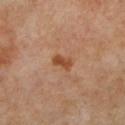Imaged during a routine full-body skin examination; the lesion was not biopsied and no histopathology is available. This is a cross-polarized tile. An algorithmic analysis of the crop reported internal color variation of about 2.5 on a 0–10 scale and a peripheral color-asymmetry measure near 0.5. The software also gave a nevus-likeness score of about 85/100 and a lesion-detection confidence of about 100/100. A female subject aged approximately 50. On the left lower leg. A roughly 15 mm field-of-view crop from a total-body skin photograph. Longest diameter approximately 2.5 mm.